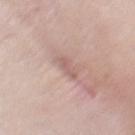workup: total-body-photography surveillance lesion; no biopsy | illumination: white-light illumination | diameter: about 2.5 mm | anatomic site: the front of the torso | patient: female, aged approximately 45 | acquisition: ~15 mm tile from a whole-body skin photo | image-analysis metrics: an area of roughly 3.5 mm² and an eccentricity of roughly 0.8.